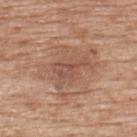Imaged during a routine full-body skin examination; the lesion was not biopsied and no histopathology is available. A roughly 15 mm field-of-view crop from a total-body skin photograph. This is a white-light tile. Located on the upper back. About 4 mm across. The subject is a male approximately 60 years of age. An algorithmic analysis of the crop reported an outline eccentricity of about 0.9 (0 = round, 1 = elongated) and two-axis asymmetry of about 0.45. And it measured a mean CIELAB color near L≈51 a*≈21 b*≈28 and a normalized border contrast of about 5.5. And it measured a border-irregularity index near 5.5/10, a within-lesion color-variation index near 3/10, and a peripheral color-asymmetry measure near 1. The software also gave a nevus-likeness score of about 0/100.